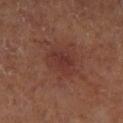Captured during whole-body skin photography for melanoma surveillance; the lesion was not biopsied.
A region of skin cropped from a whole-body photographic capture, roughly 15 mm wide.
The lesion is located on the right lower leg.
Longest diameter approximately 3.5 mm.
Imaged with cross-polarized lighting.
A female patient.